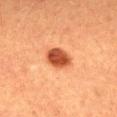Assessment:
Imaged during a routine full-body skin examination; the lesion was not biopsied and no histopathology is available.
Acquisition and patient details:
The patient is a male about 40 years old. From the mid back. An algorithmic analysis of the crop reported a mean CIELAB color near L≈44 a*≈29 b*≈35, roughly 15 lightness units darker than nearby skin, and a lesion-to-skin contrast of about 11 (normalized; higher = more distinct). The software also gave a border-irregularity rating of about 1.5/10, internal color variation of about 3.5 on a 0–10 scale, and radial color variation of about 1. The analysis additionally found lesion-presence confidence of about 100/100. Approximately 3.5 mm at its widest. A close-up tile cropped from a whole-body skin photograph, about 15 mm across.